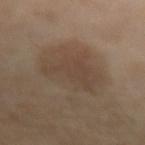<record>
  <biopsy_status>not biopsied; imaged during a skin examination</biopsy_status>
  <site>left forearm</site>
  <lighting>cross-polarized</lighting>
  <lesion_size>
    <long_diameter_mm_approx>7.5</long_diameter_mm_approx>
  </lesion_size>
  <image>
    <source>total-body photography crop</source>
    <field_of_view_mm>15</field_of_view_mm>
  </image>
  <patient>
    <sex>female</sex>
    <age_approx>45</age_approx>
  </patient>
</record>